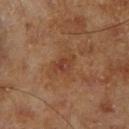| feature | finding |
|---|---|
| workup | imaged on a skin check; not biopsied |